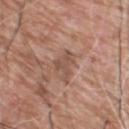Clinical impression:
This lesion was catalogued during total-body skin photography and was not selected for biopsy.
Image and clinical context:
The lesion's longest dimension is about 3 mm. A male patient aged 58–62. Imaged with white-light lighting. Cropped from a whole-body photographic skin survey; the tile spans about 15 mm. The lesion-visualizer software estimated an area of roughly 4.5 mm² and a shape eccentricity near 0.5. Located on the upper back.